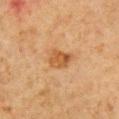notes=no biopsy performed (imaged during a skin exam) | tile lighting=cross-polarized | TBP lesion metrics=a lesion color around L≈46 a*≈20 b*≈36 in CIELAB and a lesion–skin lightness drop of about 9; a border-irregularity rating of about 2.5/10, internal color variation of about 3.5 on a 0–10 scale, and peripheral color asymmetry of about 1.5; lesion-presence confidence of about 100/100 | anatomic site=the right upper arm | image=total-body-photography crop, ~15 mm field of view | subject=male, roughly 60 years of age | diameter=~3 mm (longest diameter).